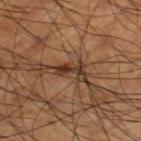Acquisition and patient details: Automated tile analysis of the lesion measured an area of roughly 3 mm², an eccentricity of roughly 0.95, and a symmetry-axis asymmetry near 0.4. And it measured a mean CIELAB color near L≈33 a*≈19 b*≈28, a lesion–skin lightness drop of about 12, and a normalized lesion–skin contrast near 11. And it measured radial color variation of about 0. The software also gave an automated nevus-likeness rating near 5 out of 100. The lesion's longest dimension is about 3.5 mm. From the left thigh. Imaged with cross-polarized lighting. A roughly 15 mm field-of-view crop from a total-body skin photograph. The subject is a male approximately 60 years of age.